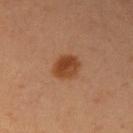This lesion was catalogued during total-body skin photography and was not selected for biopsy. A female patient, aged around 30. The lesion is on the right upper arm. The recorded lesion diameter is about 3.5 mm. A roughly 15 mm field-of-view crop from a total-body skin photograph. This is a cross-polarized tile.